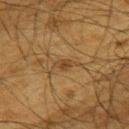Q: Lesion location?
A: the upper back
Q: Lesion size?
A: ≈2.5 mm
Q: What are the patient's age and sex?
A: male, about 65 years old
Q: What kind of image is this?
A: ~15 mm tile from a whole-body skin photo
Q: Automated lesion metrics?
A: a lesion area of about 3 mm², an eccentricity of roughly 0.85, and a symmetry-axis asymmetry near 0.35; a lesion color around L≈35 a*≈15 b*≈31 in CIELAB, about 7 CIELAB-L* units darker than the surrounding skin, and a normalized border contrast of about 6.5; a color-variation rating of about 1.5/10 and peripheral color asymmetry of about 0.5